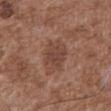| field | value |
|---|---|
| biopsy status | no biopsy performed (imaged during a skin exam) |
| imaging modality | 15 mm crop, total-body photography |
| lesion diameter | ≈4 mm |
| patient | male, in their mid- to late 70s |
| site | the chest |